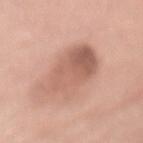Recorded during total-body skin imaging; not selected for excision or biopsy. On the abdomen. This is a white-light tile. Measured at roughly 9.5 mm in maximum diameter. The patient is a female roughly 65 years of age. The lesion-visualizer software estimated an area of roughly 23 mm², a shape eccentricity near 0.95, and a shape-asymmetry score of about 0.2 (0 = symmetric). The software also gave a border-irregularity index near 4/10, internal color variation of about 7 on a 0–10 scale, and a peripheral color-asymmetry measure near 2.5. A 15 mm close-up tile from a total-body photography series done for melanoma screening.